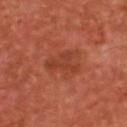Clinical summary:
From the upper back. Imaged with cross-polarized lighting. A roughly 15 mm field-of-view crop from a total-body skin photograph. Longest diameter approximately 4.5 mm. An algorithmic analysis of the crop reported two-axis asymmetry of about 0.65. The software also gave a classifier nevus-likeness of about 50/100 and a detector confidence of about 100 out of 100 that the crop contains a lesion. The patient is a male aged approximately 50.